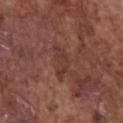Clinical summary: Approximately 4 mm at its widest. On the chest. Automated image analysis of the tile measured an average lesion color of about L≈36 a*≈21 b*≈23 (CIELAB), a lesion–skin lightness drop of about 6, and a lesion-to-skin contrast of about 5 (normalized; higher = more distinct). The analysis additionally found a classifier nevus-likeness of about 0/100 and lesion-presence confidence of about 85/100. A roughly 15 mm field-of-view crop from a total-body skin photograph. Captured under white-light illumination. A male patient, aged 73–77.